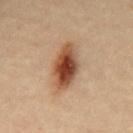• notes — no biopsy performed (imaged during a skin exam)